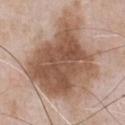The lesion was tiled from a total-body skin photograph and was not biopsied. Located on the front of the torso. Automated tile analysis of the lesion measured a mean CIELAB color near L≈53 a*≈19 b*≈29 and a normalized border contrast of about 10. This is a white-light tile. A male patient aged 48 to 52. Measured at roughly 9.5 mm in maximum diameter. A 15 mm close-up tile from a total-body photography series done for melanoma screening.